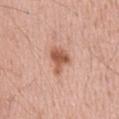This lesion was catalogued during total-body skin photography and was not selected for biopsy.
A lesion tile, about 15 mm wide, cut from a 3D total-body photograph.
On the abdomen.
The subject is a male aged around 60.